The subject is a female roughly 35 years of age; a close-up tile cropped from a whole-body skin photograph, about 15 mm across; captured under cross-polarized illumination; the lesion's longest dimension is about 2 mm; on the leg: 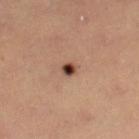On excision, pathology confirmed an atypical melanocytic neoplasm — a lesion of indeterminate malignant potential.